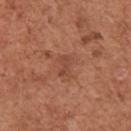Impression:
This lesion was catalogued during total-body skin photography and was not selected for biopsy.
Image and clinical context:
Automated image analysis of the tile measured a lesion area of about 3.5 mm² and a symmetry-axis asymmetry near 0.25. And it measured a border-irregularity index near 3.5/10, internal color variation of about 1 on a 0–10 scale, and peripheral color asymmetry of about 0.5. Cropped from a whole-body photographic skin survey; the tile spans about 15 mm. The lesion's longest dimension is about 2.5 mm. Captured under white-light illumination. A female patient, in their 50s. On the right upper arm.